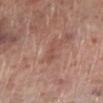<record>
<biopsy_status>not biopsied; imaged during a skin examination</biopsy_status>
<patient>
  <sex>male</sex>
  <age_approx>70</age_approx>
</patient>
<lighting>white-light</lighting>
<site>left lower leg</site>
<image>
  <source>total-body photography crop</source>
  <field_of_view_mm>15</field_of_view_mm>
</image>
</record>